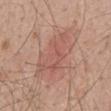| key | value |
|---|---|
| workup | no biopsy performed (imaged during a skin exam) |
| subject | male, aged 48–52 |
| site | the mid back |
| imaging modality | total-body-photography crop, ~15 mm field of view |